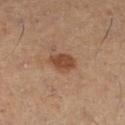Captured during whole-body skin photography for melanoma surveillance; the lesion was not biopsied.
The patient is a female aged approximately 50.
From the right lower leg.
A 15 mm close-up tile from a total-body photography series done for melanoma screening.
The total-body-photography lesion software estimated a lesion color around L≈45 a*≈20 b*≈31 in CIELAB, a lesion–skin lightness drop of about 10, and a normalized lesion–skin contrast near 8. It also reported a classifier nevus-likeness of about 95/100.
Approximately 3.5 mm at its widest.
The tile uses cross-polarized illumination.